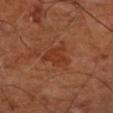No biopsy was performed on this lesion — it was imaged during a full skin examination and was not determined to be concerning.
Cropped from a total-body skin-imaging series; the visible field is about 15 mm.
A patient about 65 years old.
On the arm.
The total-body-photography lesion software estimated an outline eccentricity of about 0.55 (0 = round, 1 = elongated). It also reported an average lesion color of about L≈36 a*≈26 b*≈33 (CIELAB) and a lesion–skin lightness drop of about 6. The analysis additionally found border irregularity of about 6 on a 0–10 scale and a color-variation rating of about 1.5/10.